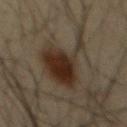| key | value |
|---|---|
| patient | male, approximately 35 years of age |
| location | the mid back |
| image | ~15 mm crop, total-body skin-cancer survey |